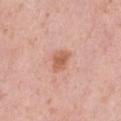Q: Is there a histopathology result?
A: total-body-photography surveillance lesion; no biopsy
Q: What kind of image is this?
A: 15 mm crop, total-body photography
Q: What is the anatomic site?
A: the left thigh
Q: Patient demographics?
A: female, aged 38 to 42
Q: Illumination type?
A: white-light illumination